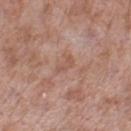Imaged during a routine full-body skin examination; the lesion was not biopsied and no histopathology is available.
A roughly 15 mm field-of-view crop from a total-body skin photograph.
The patient is a male about 55 years old.
Longest diameter approximately 2.5 mm.
From the right lower leg.